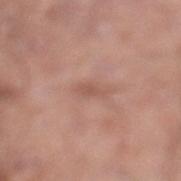body site = the left lower leg; illumination = white-light; subject = male, roughly 20 years of age; imaging modality = total-body-photography crop, ~15 mm field of view; diameter = ≈2.5 mm.